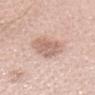Findings:
• workup · total-body-photography surveillance lesion; no biopsy
• illumination · white-light
• image-analysis metrics · a shape eccentricity near 0.75 and two-axis asymmetry of about 0.2; border irregularity of about 2 on a 0–10 scale, a color-variation rating of about 3/10, and a peripheral color-asymmetry measure near 1
• diameter · about 4.5 mm
• anatomic site · the head or neck
• subject · female, roughly 25 years of age
• acquisition · ~15 mm crop, total-body skin-cancer survey